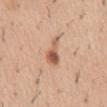Case summary:
• notes · no biopsy performed (imaged during a skin exam)
• imaging modality · total-body-photography crop, ~15 mm field of view
• patient · male, aged around 40
• lighting · white-light
• automated lesion analysis · an average lesion color of about L≈58 a*≈22 b*≈32 (CIELAB), a lesion–skin lightness drop of about 13, and a lesion-to-skin contrast of about 8 (normalized; higher = more distinct); border irregularity of about 5 on a 0–10 scale and internal color variation of about 5.5 on a 0–10 scale
• body site · the front of the torso
• lesion size · ~3.5 mm (longest diameter)